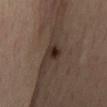workup: catalogued during a skin exam; not biopsied
acquisition: 15 mm crop, total-body photography
subject: male, approximately 45 years of age
body site: the mid back
image-analysis metrics: a footprint of about 5 mm² and a shape eccentricity near 0.7; an average lesion color of about L≈28 a*≈14 b*≈20 (CIELAB), a lesion–skin lightness drop of about 10, and a lesion-to-skin contrast of about 10.5 (normalized; higher = more distinct); border irregularity of about 3 on a 0–10 scale, internal color variation of about 5.5 on a 0–10 scale, and a peripheral color-asymmetry measure near 2; a classifier nevus-likeness of about 100/100 and a detector confidence of about 100 out of 100 that the crop contains a lesion